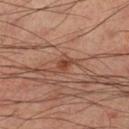Imaged during a routine full-body skin examination; the lesion was not biopsied and no histopathology is available.
Imaged with cross-polarized lighting.
The lesion is located on the left lower leg.
A region of skin cropped from a whole-body photographic capture, roughly 15 mm wide.
A male subject, approximately 45 years of age.
The total-body-photography lesion software estimated about 8 CIELAB-L* units darker than the surrounding skin and a normalized border contrast of about 7.